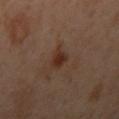This lesion was catalogued during total-body skin photography and was not selected for biopsy. Automated tile analysis of the lesion measured an eccentricity of roughly 0.5 and a symmetry-axis asymmetry near 0.35. And it measured a lesion color around L≈31 a*≈19 b*≈25 in CIELAB, about 8 CIELAB-L* units darker than the surrounding skin, and a normalized border contrast of about 8. It also reported a classifier nevus-likeness of about 60/100 and lesion-presence confidence of about 100/100. A female patient, aged 38 to 42. The lesion is on the left arm. A roughly 15 mm field-of-view crop from a total-body skin photograph. About 2.5 mm across.